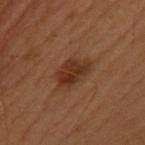Recorded during total-body skin imaging; not selected for excision or biopsy.
Cropped from a total-body skin-imaging series; the visible field is about 15 mm.
The lesion-visualizer software estimated a shape eccentricity near 0.75 and a symmetry-axis asymmetry near 0.25. The analysis additionally found a mean CIELAB color near L≈27 a*≈19 b*≈26, about 8 CIELAB-L* units darker than the surrounding skin, and a lesion-to-skin contrast of about 9 (normalized; higher = more distinct). The software also gave a border-irregularity rating of about 2.5/10, a within-lesion color-variation index near 3/10, and radial color variation of about 1. And it measured an automated nevus-likeness rating near 75 out of 100 and lesion-presence confidence of about 100/100.
A female subject aged around 50.
Imaged with cross-polarized lighting.
On the upper back.
Measured at roughly 4 mm in maximum diameter.